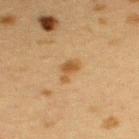Case summary:
- notes · total-body-photography surveillance lesion; no biopsy
- tile lighting · cross-polarized illumination
- body site · the upper back
- image-analysis metrics · a lesion area of about 4 mm², a shape eccentricity near 0.85, and a shape-asymmetry score of about 0.5 (0 = symmetric)
- subject · female, aged approximately 40
- imaging modality · 15 mm crop, total-body photography